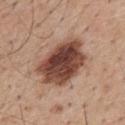Clinical impression: Captured during whole-body skin photography for melanoma surveillance; the lesion was not biopsied. Background: A male subject in their mid-50s. Longest diameter approximately 6.5 mm. This is a white-light tile. The lesion is on the mid back. A region of skin cropped from a whole-body photographic capture, roughly 15 mm wide.